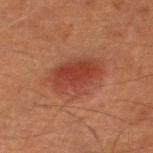Imaged during a routine full-body skin examination; the lesion was not biopsied and no histopathology is available. An algorithmic analysis of the crop reported a footprint of about 19 mm² and an eccentricity of roughly 0.75. It also reported a mean CIELAB color near L≈34 a*≈23 b*≈26, about 7 CIELAB-L* units darker than the surrounding skin, and a lesion-to-skin contrast of about 7 (normalized; higher = more distinct). And it measured a border-irregularity rating of about 2/10, a within-lesion color-variation index near 4/10, and a peripheral color-asymmetry measure near 1. It also reported a nevus-likeness score of about 100/100 and lesion-presence confidence of about 100/100. A male patient, in their mid- to late 60s. The lesion is located on the leg. Captured under cross-polarized illumination. Longest diameter approximately 6.5 mm. This image is a 15 mm lesion crop taken from a total-body photograph.